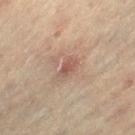notes: imaged on a skin check; not biopsied.